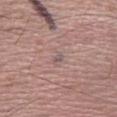Q: Where on the body is the lesion?
A: the left forearm
Q: Patient demographics?
A: male, aged around 35
Q: What is the imaging modality?
A: 15 mm crop, total-body photography
Q: What did automated image analysis measure?
A: border irregularity of about 3 on a 0–10 scale, a color-variation rating of about 0/10, and a peripheral color-asymmetry measure near 0
Q: How was the tile lit?
A: white-light